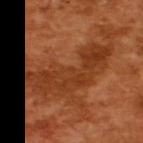workup = no biopsy performed (imaged during a skin exam)
image-analysis metrics = a mean CIELAB color near L≈36 a*≈27 b*≈37 and about 8 CIELAB-L* units darker than the surrounding skin; border irregularity of about 9 on a 0–10 scale, internal color variation of about 3.5 on a 0–10 scale, and radial color variation of about 1
diameter = ≈9.5 mm
imaging modality = 15 mm crop, total-body photography
patient = male, roughly 65 years of age
tile lighting = cross-polarized illumination